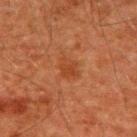Recorded during total-body skin imaging; not selected for excision or biopsy. The lesion is on the upper back. Automated image analysis of the tile measured a footprint of about 5 mm², an outline eccentricity of about 0.7 (0 = round, 1 = elongated), and two-axis asymmetry of about 0.3. The software also gave a color-variation rating of about 2.5/10 and radial color variation of about 1. It also reported a classifier nevus-likeness of about 0/100 and a detector confidence of about 100 out of 100 that the crop contains a lesion. A male subject aged 58 to 62. A 15 mm close-up tile from a total-body photography series done for melanoma screening.